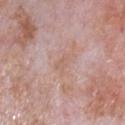No biopsy was performed on this lesion — it was imaged during a full skin examination and was not determined to be concerning. On the chest. A male subject, about 65 years old. Approximately 2.5 mm at its widest. A region of skin cropped from a whole-body photographic capture, roughly 15 mm wide. This is a white-light tile. The total-body-photography lesion software estimated a border-irregularity rating of about 3.5/10, internal color variation of about 0 on a 0–10 scale, and a peripheral color-asymmetry measure near 0. And it measured an automated nevus-likeness rating near 0 out of 100 and lesion-presence confidence of about 100/100.